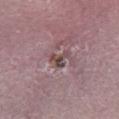Part of a total-body skin-imaging series; this lesion was reviewed on a skin check and was not flagged for biopsy. The patient is a male aged approximately 55. The recorded lesion diameter is about 2.5 mm. The tile uses white-light illumination. A roughly 15 mm field-of-view crop from a total-body skin photograph. The lesion-visualizer software estimated a mean CIELAB color near L≈45 a*≈17 b*≈17, roughly 11 lightness units darker than nearby skin, and a normalized lesion–skin contrast near 8.5. The lesion is located on the right lower leg.